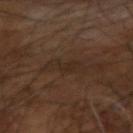Clinical impression:
Imaged during a routine full-body skin examination; the lesion was not biopsied and no histopathology is available.
Acquisition and patient details:
On the left arm. This image is a 15 mm lesion crop taken from a total-body photograph. Imaged with cross-polarized lighting. A male patient approximately 60 years of age. The lesion-visualizer software estimated roughly 4 lightness units darker than nearby skin and a normalized border contrast of about 5. And it measured a border-irregularity rating of about 6/10, internal color variation of about 2 on a 0–10 scale, and peripheral color asymmetry of about 1. It also reported a detector confidence of about 60 out of 100 that the crop contains a lesion. About 4 mm across.